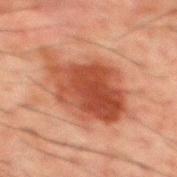body site=the mid back
acquisition=~15 mm crop, total-body skin-cancer survey
subject=male, aged approximately 50